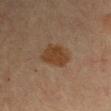Image and clinical context: About 4 mm across. A male subject, approximately 60 years of age. From the left upper arm. Cropped from a total-body skin-imaging series; the visible field is about 15 mm.